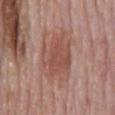notes = total-body-photography surveillance lesion; no biopsy
image source = ~15 mm crop, total-body skin-cancer survey
lesion diameter = about 7 mm
subject = male, roughly 75 years of age
lighting = white-light illumination
body site = the back